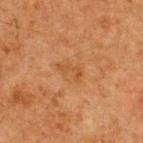Notes:
- workup: no biopsy performed (imaged during a skin exam)
- subject: male, in their mid-60s
- anatomic site: the upper back
- image source: ~15 mm crop, total-body skin-cancer survey
- automated lesion analysis: a mean CIELAB color near L≈43 a*≈22 b*≈36 and roughly 6 lightness units darker than nearby skin; a border-irregularity index near 6.5/10, a within-lesion color-variation index near 0/10, and radial color variation of about 0